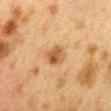Assessment:
This lesion was catalogued during total-body skin photography and was not selected for biopsy.
Clinical summary:
A female subject in their 40s. Cropped from a total-body skin-imaging series; the visible field is about 15 mm. Longest diameter approximately 2.5 mm. Automated tile analysis of the lesion measured border irregularity of about 2 on a 0–10 scale, a color-variation rating of about 4.5/10, and a peripheral color-asymmetry measure near 1.5. The analysis additionally found a classifier nevus-likeness of about 75/100 and lesion-presence confidence of about 100/100. The lesion is on the mid back. This is a cross-polarized tile.